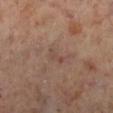workup = catalogued during a skin exam; not biopsied
imaging modality = total-body-photography crop, ~15 mm field of view
automated metrics = a mean CIELAB color near L≈45 a*≈18 b*≈24, about 6 CIELAB-L* units darker than the surrounding skin, and a normalized lesion–skin contrast near 4.5; an automated nevus-likeness rating near 0 out of 100
illumination = cross-polarized illumination
lesion size = ~2.5 mm (longest diameter)
patient = female, approximately 60 years of age
body site = the right lower leg